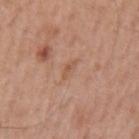A male patient about 60 years old. Imaged with white-light lighting. Automated tile analysis of the lesion measured a lesion area of about 2.5 mm² and a symmetry-axis asymmetry near 0.25. And it measured a lesion color around L≈55 a*≈21 b*≈32 in CIELAB, roughly 6 lightness units darker than nearby skin, and a lesion-to-skin contrast of about 5 (normalized; higher = more distinct). The software also gave internal color variation of about 0.5 on a 0–10 scale and peripheral color asymmetry of about 0. A 15 mm close-up extracted from a 3D total-body photography capture. The lesion is located on the left upper arm.